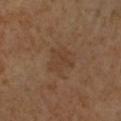Impression: Imaged during a routine full-body skin examination; the lesion was not biopsied and no histopathology is available. Context: The lesion is on the right upper arm. This is a cross-polarized tile. A female subject, aged around 55. This image is a 15 mm lesion crop taken from a total-body photograph. Approximately 4 mm at its widest.